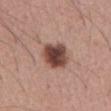Located on the abdomen.
A male subject, in their mid- to late 50s.
A 15 mm crop from a total-body photograph taken for skin-cancer surveillance.
Longest diameter approximately 3.5 mm.